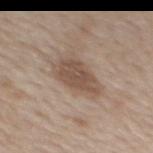site: the mid back; acquisition: ~15 mm crop, total-body skin-cancer survey; patient: male, aged around 60.